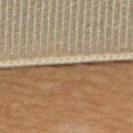notes — imaged on a skin check; not biopsied | lesion diameter — ~2.5 mm (longest diameter) | illumination — cross-polarized | acquisition — total-body-photography crop, ~15 mm field of view | patient — female, aged around 65 | anatomic site — the upper back | automated lesion analysis — an outline eccentricity of about 0.95 (0 = round, 1 = elongated) and two-axis asymmetry of about 0.35; a border-irregularity rating of about 4/10, a color-variation rating of about 0/10, and radial color variation of about 0; an automated nevus-likeness rating near 0 out of 100.